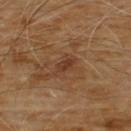Impression:
Captured during whole-body skin photography for melanoma surveillance; the lesion was not biopsied.
Image and clinical context:
A roughly 15 mm field-of-view crop from a total-body skin photograph. The subject is a male aged approximately 60. This is a cross-polarized tile. The lesion is on the chest.